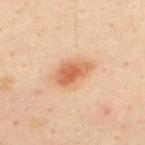biopsy_status: not biopsied; imaged during a skin examination
lesion_size:
  long_diameter_mm_approx: 4.0
site: upper back
lighting: cross-polarized
image:
  source: total-body photography crop
  field_of_view_mm: 15
patient:
  sex: male
  age_approx: 50